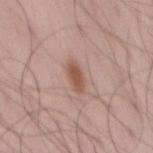biopsy status — catalogued during a skin exam; not biopsied | patient — male, aged around 45 | location — the mid back | image — ~15 mm crop, total-body skin-cancer survey | illumination — white-light.